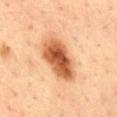{"biopsy_status": "not biopsied; imaged during a skin examination", "patient": {"sex": "male", "age_approx": 35}, "lighting": "cross-polarized", "lesion_size": {"long_diameter_mm_approx": 6.0}, "automated_metrics": {"vs_skin_darker_L": 18.0}, "image": {"source": "total-body photography crop", "field_of_view_mm": 15}, "site": "mid back"}